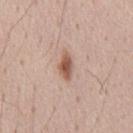This lesion was catalogued during total-body skin photography and was not selected for biopsy. A close-up tile cropped from a whole-body skin photograph, about 15 mm across. Automated image analysis of the tile measured a border-irregularity rating of about 2.5/10, a color-variation rating of about 3/10, and a peripheral color-asymmetry measure near 0.5. The analysis additionally found a nevus-likeness score of about 100/100 and a lesion-detection confidence of about 100/100. From the chest. The recorded lesion diameter is about 3.5 mm. The subject is a male aged 43 to 47.